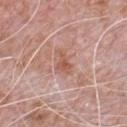biopsy status = catalogued during a skin exam; not biopsied
image = ~15 mm tile from a whole-body skin photo
location = the chest
patient = male, aged around 80
diameter = ≈2.5 mm